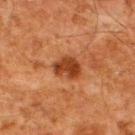No biopsy was performed on this lesion — it was imaged during a full skin examination and was not determined to be concerning. A male subject aged 58 to 62. An algorithmic analysis of the crop reported a shape eccentricity near 0.75 and two-axis asymmetry of about 0.3. And it measured a border-irregularity rating of about 3/10, a within-lesion color-variation index near 3/10, and peripheral color asymmetry of about 1. And it measured a lesion-detection confidence of about 100/100. The lesion is on the upper back. A 15 mm close-up extracted from a 3D total-body photography capture. Longest diameter approximately 3.5 mm. Captured under cross-polarized illumination.